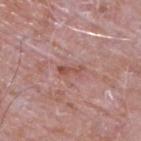This lesion was catalogued during total-body skin photography and was not selected for biopsy. A male patient, roughly 65 years of age. Automated image analysis of the tile measured a footprint of about 3 mm² and an outline eccentricity of about 0.95 (0 = round, 1 = elongated). The software also gave a nevus-likeness score of about 0/100 and a lesion-detection confidence of about 95/100. A roughly 15 mm field-of-view crop from a total-body skin photograph. The tile uses white-light illumination. The lesion is on the back. The recorded lesion diameter is about 3 mm.